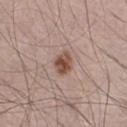Imaged during a routine full-body skin examination; the lesion was not biopsied and no histopathology is available.
A close-up tile cropped from a whole-body skin photograph, about 15 mm across.
The recorded lesion diameter is about 3 mm.
The patient is a male in their 30s.